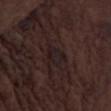Clinical impression: No biopsy was performed on this lesion — it was imaged during a full skin examination and was not determined to be concerning. Background: A male patient, aged approximately 75. Automated image analysis of the tile measured an average lesion color of about L≈17 a*≈12 b*≈11 (CIELAB), a lesion–skin lightness drop of about 4, and a lesion-to-skin contrast of about 7.5 (normalized; higher = more distinct). The analysis additionally found a border-irregularity rating of about 2.5/10 and internal color variation of about 2.5 on a 0–10 scale. About 2.5 mm across. Located on the left thigh. A 15 mm close-up extracted from a 3D total-body photography capture.